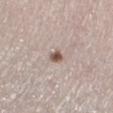{"biopsy_status": "not biopsied; imaged during a skin examination", "patient": {"sex": "female", "age_approx": 55}, "site": "right lower leg", "image": {"source": "total-body photography crop", "field_of_view_mm": 15}}